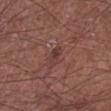The lesion was photographed on a routine skin check and not biopsied; there is no pathology result.
The lesion is on the left lower leg.
A male patient, roughly 65 years of age.
A roughly 15 mm field-of-view crop from a total-body skin photograph.
The tile uses white-light illumination.
The lesion's longest dimension is about 2.5 mm.